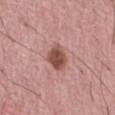* biopsy status · catalogued during a skin exam; not biopsied
* size · about 3 mm
* automated metrics · an area of roughly 7 mm², an eccentricity of roughly 0.6, and a shape-asymmetry score of about 0.25 (0 = symmetric); a border-irregularity rating of about 2/10, a within-lesion color-variation index near 4/10, and peripheral color asymmetry of about 1.5
* subject · male, approximately 50 years of age
* illumination · white-light
* body site · the front of the torso
* acquisition · ~15 mm tile from a whole-body skin photo